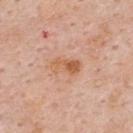  site: upper back
  lighting: white-light
  patient:
    sex: male
    age_approx: 60
  lesion_size:
    long_diameter_mm_approx: 3.5
  image:
    source: total-body photography crop
    field_of_view_mm: 15